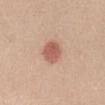biopsy_status: not biopsied; imaged during a skin examination
lesion_size:
  long_diameter_mm_approx: 3.0
patient:
  sex: female
  age_approx: 50
lighting: white-light
site: abdomen
image:
  source: total-body photography crop
  field_of_view_mm: 15